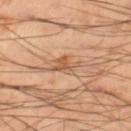This lesion was catalogued during total-body skin photography and was not selected for biopsy.
From the right lower leg.
Longest diameter approximately 3 mm.
A male patient, aged 48–52.
The tile uses cross-polarized illumination.
The total-body-photography lesion software estimated an average lesion color of about L≈56 a*≈22 b*≈35 (CIELAB), roughly 10 lightness units darker than nearby skin, and a lesion-to-skin contrast of about 6.5 (normalized; higher = more distinct). The analysis additionally found a classifier nevus-likeness of about 5/100 and a lesion-detection confidence of about 100/100.
A close-up tile cropped from a whole-body skin photograph, about 15 mm across.